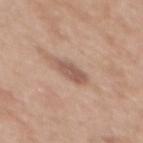Impression:
The lesion was photographed on a routine skin check and not biopsied; there is no pathology result.
Clinical summary:
The subject is a female aged 38 to 42. From the mid back. A 15 mm close-up tile from a total-body photography series done for melanoma screening. Imaged with white-light lighting.